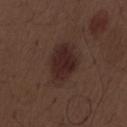<lesion>
  <biopsy_status>not biopsied; imaged during a skin examination</biopsy_status>
  <automated_metrics>
    <area_mm2_approx>15.0</area_mm2_approx>
    <eccentricity>0.75</eccentricity>
    <shape_asymmetry>0.2</shape_asymmetry>
    <cielab_L>25</cielab_L>
    <cielab_a>16</cielab_a>
    <cielab_b>18</cielab_b>
    <vs_skin_contrast_norm>9.0</vs_skin_contrast_norm>
    <border_irregularity_0_10>2.0</border_irregularity_0_10>
    <color_variation_0_10>3.0</color_variation_0_10>
  </automated_metrics>
  <lesion_size>
    <long_diameter_mm_approx>5.5</long_diameter_mm_approx>
  </lesion_size>
  <patient>
    <sex>male</sex>
    <age_approx>70</age_approx>
  </patient>
  <lighting>white-light</lighting>
  <site>abdomen</site>
  <image>
    <source>total-body photography crop</source>
    <field_of_view_mm>15</field_of_view_mm>
  </image>
</lesion>